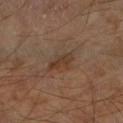{
  "biopsy_status": "not biopsied; imaged during a skin examination",
  "site": "right forearm",
  "lesion_size": {
    "long_diameter_mm_approx": 3.5
  },
  "lighting": "cross-polarized",
  "patient": {
    "sex": "male",
    "age_approx": 70
  },
  "image": {
    "source": "total-body photography crop",
    "field_of_view_mm": 15
  }
}